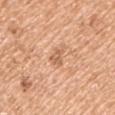Case summary:
* notes · total-body-photography surveillance lesion; no biopsy
* body site · the left upper arm
* acquisition · ~15 mm tile from a whole-body skin photo
* subject · male, aged 53–57
* automated metrics · an area of roughly 3.5 mm², an outline eccentricity of about 0.75 (0 = round, 1 = elongated), and a symmetry-axis asymmetry near 0.35; a normalized border contrast of about 6
* lesion diameter · ≈2.5 mm